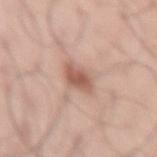Part of a total-body skin-imaging series; this lesion was reviewed on a skin check and was not flagged for biopsy. The recorded lesion diameter is about 3 mm. An algorithmic analysis of the crop reported lesion-presence confidence of about 100/100. This is a white-light tile. The lesion is located on the lower back. Cropped from a total-body skin-imaging series; the visible field is about 15 mm. The subject is a male aged 43 to 47.